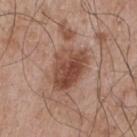follow-up — total-body-photography surveillance lesion; no biopsy | lighting — white-light illumination | body site — the chest | subject — male, aged 63–67 | diameter — ≈5 mm | image — 15 mm crop, total-body photography.